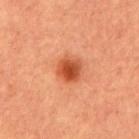Longest diameter approximately 3 mm. The tile uses cross-polarized illumination. A roughly 15 mm field-of-view crop from a total-body skin photograph. The subject is a male approximately 65 years of age. The lesion is located on the abdomen.